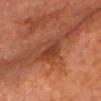biopsy status: no biopsy performed (imaged during a skin exam)
lighting: cross-polarized
automated lesion analysis: two-axis asymmetry of about 0.35; a lesion color around L≈33 a*≈22 b*≈27 in CIELAB, roughly 7 lightness units darker than nearby skin, and a lesion-to-skin contrast of about 7 (normalized; higher = more distinct); an automated nevus-likeness rating near 0 out of 100 and a lesion-detection confidence of about 75/100
patient: male, roughly 75 years of age
image: total-body-photography crop, ~15 mm field of view
diameter: ~4.5 mm (longest diameter)
body site: the chest